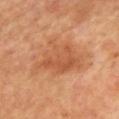  lesion_size:
    long_diameter_mm_approx: 7.0
  lighting: cross-polarized
  site: mid back
  image:
    source: total-body photography crop
    field_of_view_mm: 15
  patient:
    age_approx: 65